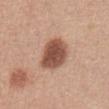<tbp_lesion>
<biopsy_status>not biopsied; imaged during a skin examination</biopsy_status>
<patient>
  <sex>female</sex>
  <age_approx>55</age_approx>
</patient>
<image>
  <source>total-body photography crop</source>
  <field_of_view_mm>15</field_of_view_mm>
</image>
<site>abdomen</site>
<lesion_size>
  <long_diameter_mm_approx>4.0</long_diameter_mm_approx>
</lesion_size>
<lighting>white-light</lighting>
</tbp_lesion>